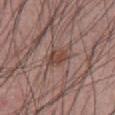• follow-up — no biopsy performed (imaged during a skin exam)
• location — the abdomen
• size — ~2.5 mm (longest diameter)
• patient — male, aged around 55
• illumination — white-light
• acquisition — 15 mm crop, total-body photography